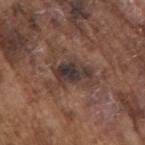{
  "biopsy_status": "not biopsied; imaged during a skin examination",
  "site": "right upper arm",
  "image": {
    "source": "total-body photography crop",
    "field_of_view_mm": 15
  },
  "lesion_size": {
    "long_diameter_mm_approx": 4.0
  },
  "patient": {
    "sex": "male",
    "age_approx": 75
  }
}